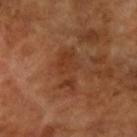Impression: The lesion was tiled from a total-body skin photograph and was not biopsied. Image and clinical context: A female subject roughly 70 years of age. Captured under cross-polarized illumination. The lesion's longest dimension is about 5.5 mm. The lesion is on the right forearm. A 15 mm close-up extracted from a 3D total-body photography capture.